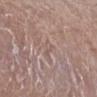* site — the right lower leg
* illumination — white-light illumination
* lesion size — about 2.5 mm
* subject — male, roughly 50 years of age
* acquisition — 15 mm crop, total-body photography